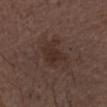workup: imaged on a skin check; not biopsied | lighting: white-light illumination | size: ≈3.5 mm | imaging modality: 15 mm crop, total-body photography | patient: male, in their mid-70s | location: the head or neck | automated metrics: a footprint of about 8.5 mm², a shape eccentricity near 0.45, and two-axis asymmetry of about 0.3; a mean CIELAB color near L≈29 a*≈15 b*≈21, roughly 6 lightness units darker than nearby skin, and a normalized border contrast of about 6.5; border irregularity of about 3.5 on a 0–10 scale, a color-variation rating of about 2/10, and peripheral color asymmetry of about 0.5; a lesion-detection confidence of about 100/100.